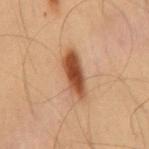On the mid back. Automated image analysis of the tile measured a lesion area of about 9 mm² and a shape eccentricity near 0.95. It also reported a border-irregularity rating of about 3/10 and internal color variation of about 4 on a 0–10 scale. Longest diameter approximately 5.5 mm. Imaged with cross-polarized lighting. The subject is a male about 55 years old. A lesion tile, about 15 mm wide, cut from a 3D total-body photograph.